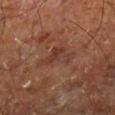Q: Is there a histopathology result?
A: catalogued during a skin exam; not biopsied
Q: What are the patient's age and sex?
A: male, about 60 years old
Q: What is the anatomic site?
A: the right leg
Q: What kind of image is this?
A: 15 mm crop, total-body photography
Q: What is the lesion's diameter?
A: about 5 mm
Q: Automated lesion metrics?
A: a lesion–skin lightness drop of about 6; a border-irregularity index near 6/10, a color-variation rating of about 5/10, and peripheral color asymmetry of about 1.5